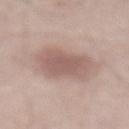Part of a total-body skin-imaging series; this lesion was reviewed on a skin check and was not flagged for biopsy. Cropped from a whole-body photographic skin survey; the tile spans about 15 mm. The patient is a male approximately 55 years of age. The lesion is located on the abdomen.